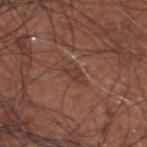Clinical impression: Part of a total-body skin-imaging series; this lesion was reviewed on a skin check and was not flagged for biopsy. Image and clinical context: The subject is a male aged approximately 65. This image is a 15 mm lesion crop taken from a total-body photograph. This is a white-light tile. The lesion is on the head or neck. Longest diameter approximately 3 mm. An algorithmic analysis of the crop reported an average lesion color of about L≈38 a*≈20 b*≈25 (CIELAB), roughly 6 lightness units darker than nearby skin, and a normalized lesion–skin contrast near 5.5. The analysis additionally found a lesion-detection confidence of about 50/100.